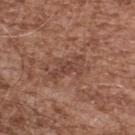<case>
<biopsy_status>not biopsied; imaged during a skin examination</biopsy_status>
<site>upper back</site>
<lighting>white-light</lighting>
<image>
  <source>total-body photography crop</source>
  <field_of_view_mm>15</field_of_view_mm>
</image>
<patient>
  <sex>male</sex>
  <age_approx>55</age_approx>
</patient>
</case>